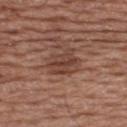lighting — white-light
location — the back
subject — male, approximately 55 years of age
lesion diameter — ≈4 mm
acquisition — ~15 mm tile from a whole-body skin photo